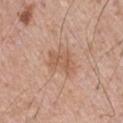follow-up — catalogued during a skin exam; not biopsied
site — the chest
subject — male, in their mid-60s
imaging modality — ~15 mm tile from a whole-body skin photo